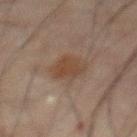Impression: Part of a total-body skin-imaging series; this lesion was reviewed on a skin check and was not flagged for biopsy.